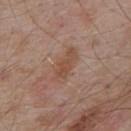Impression: This lesion was catalogued during total-body skin photography and was not selected for biopsy. Context: A male subject, aged 53–57. Automated tile analysis of the lesion measured an area of roughly 7 mm², an outline eccentricity of about 0.9 (0 = round, 1 = elongated), and two-axis asymmetry of about 0.3. The software also gave an average lesion color of about L≈49 a*≈19 b*≈29 (CIELAB) and a normalized border contrast of about 6.5. The software also gave a border-irregularity rating of about 3.5/10 and a color-variation rating of about 2/10. And it measured a nevus-likeness score of about 0/100 and a detector confidence of about 100 out of 100 that the crop contains a lesion. On the upper back. Measured at roughly 4.5 mm in maximum diameter. The tile uses white-light illumination. A roughly 15 mm field-of-view crop from a total-body skin photograph.